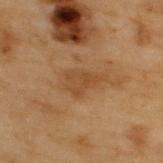Q: Was this lesion biopsied?
A: imaged on a skin check; not biopsied
Q: What are the patient's age and sex?
A: male, roughly 55 years of age
Q: Where on the body is the lesion?
A: the upper back
Q: Automated lesion metrics?
A: internal color variation of about 2 on a 0–10 scale and a peripheral color-asymmetry measure near 0.5
Q: What kind of image is this?
A: 15 mm crop, total-body photography